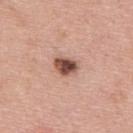A male patient, aged 38–42. Located on the back. A close-up tile cropped from a whole-body skin photograph, about 15 mm across. The tile uses white-light illumination. An algorithmic analysis of the crop reported a normalized border contrast of about 12. The analysis additionally found a classifier nevus-likeness of about 85/100 and a detector confidence of about 100 out of 100 that the crop contains a lesion. The lesion was biopsied, and histopathology showed a dysplastic (Clark) nevus.